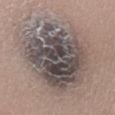No biopsy was performed on this lesion — it was imaged during a full skin examination and was not determined to be concerning. A female patient, in their mid- to late 30s. A close-up tile cropped from a whole-body skin photograph, about 15 mm across. An algorithmic analysis of the crop reported an area of roughly 65 mm² and a symmetry-axis asymmetry near 0.2. The software also gave roughly 13 lightness units darker than nearby skin and a normalized border contrast of about 10.5. The analysis additionally found internal color variation of about 9.5 on a 0–10 scale and radial color variation of about 3. And it measured an automated nevus-likeness rating near 10 out of 100 and a lesion-detection confidence of about 25/100. The tile uses white-light illumination. The lesion is on the left lower leg.